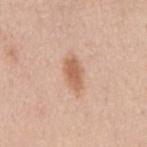A male patient, about 50 years old.
This is a white-light tile.
A region of skin cropped from a whole-body photographic capture, roughly 15 mm wide.
About 4 mm across.
The lesion is located on the mid back.